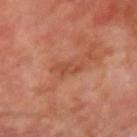workup: catalogued during a skin exam; not biopsied
diameter: about 3 mm
anatomic site: the left upper arm
imaging modality: total-body-photography crop, ~15 mm field of view
subject: male, about 70 years old